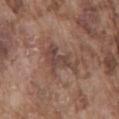* workup · no biopsy performed (imaged during a skin exam)
* imaging modality · ~15 mm crop, total-body skin-cancer survey
* TBP lesion metrics · a shape-asymmetry score of about 0.5 (0 = symmetric); an average lesion color of about L≈44 a*≈18 b*≈23 (CIELAB), a lesion–skin lightness drop of about 8, and a normalized lesion–skin contrast near 6.5; a border-irregularity rating of about 7.5/10, internal color variation of about 2.5 on a 0–10 scale, and radial color variation of about 0.5; an automated nevus-likeness rating near 0 out of 100 and a lesion-detection confidence of about 90/100
* patient · male, in their mid-70s
* lesion size · ≈5 mm
* site · the mid back
* illumination · white-light